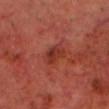This lesion was catalogued during total-body skin photography and was not selected for biopsy.
Imaged with cross-polarized lighting.
A male patient, aged around 70.
Located on the head or neck.
A close-up tile cropped from a whole-body skin photograph, about 15 mm across.